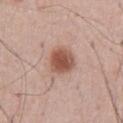Q: Is there a histopathology result?
A: total-body-photography surveillance lesion; no biopsy
Q: Lesion location?
A: the front of the torso
Q: Lesion size?
A: about 3.5 mm
Q: What kind of image is this?
A: 15 mm crop, total-body photography
Q: What are the patient's age and sex?
A: male, aged 53–57
Q: Automated lesion metrics?
A: about 13 CIELAB-L* units darker than the surrounding skin